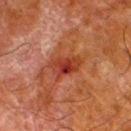follow-up: no biopsy performed (imaged during a skin exam)
lesion size: ≈4 mm
image: ~15 mm tile from a whole-body skin photo
tile lighting: cross-polarized
anatomic site: the leg
subject: male, about 80 years old